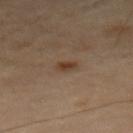| field | value |
|---|---|
| imaging modality | ~15 mm crop, total-body skin-cancer survey |
| image-analysis metrics | an outline eccentricity of about 0.7 (0 = round, 1 = elongated) and two-axis asymmetry of about 0.2; a lesion color around L≈37 a*≈16 b*≈28 in CIELAB, roughly 9 lightness units darker than nearby skin, and a normalized lesion–skin contrast near 8.5; a border-irregularity index near 1.5/10, a within-lesion color-variation index near 1.5/10, and peripheral color asymmetry of about 0.5 |
| patient | male, aged 68 to 72 |
| lighting | cross-polarized |
| body site | the leg |
| lesion size | ~2 mm (longest diameter) |